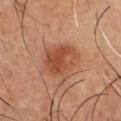This lesion was catalogued during total-body skin photography and was not selected for biopsy.
This is a cross-polarized tile.
About 4.5 mm across.
Cropped from a whole-body photographic skin survey; the tile spans about 15 mm.
On the chest.
The subject is a male approximately 55 years of age.
The lesion-visualizer software estimated a footprint of about 15 mm², an outline eccentricity of about 0.3 (0 = round, 1 = elongated), and two-axis asymmetry of about 0.25. And it measured a lesion–skin lightness drop of about 9 and a normalized border contrast of about 7. And it measured internal color variation of about 5 on a 0–10 scale and radial color variation of about 2. It also reported lesion-presence confidence of about 100/100.